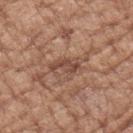Impression: The lesion was photographed on a routine skin check and not biopsied; there is no pathology result. Acquisition and patient details: A female patient, approximately 75 years of age. Located on the left upper arm. A region of skin cropped from a whole-body photographic capture, roughly 15 mm wide.